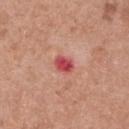workup: total-body-photography surveillance lesion; no biopsy | automated metrics: roughly 14 lightness units darker than nearby skin and a normalized lesion–skin contrast near 9.5; a border-irregularity index near 1.5/10, internal color variation of about 3 on a 0–10 scale, and radial color variation of about 1; a nevus-likeness score of about 0/100 and a detector confidence of about 100 out of 100 that the crop contains a lesion | image: ~15 mm tile from a whole-body skin photo | subject: female, roughly 60 years of age | size: ≈2.5 mm | site: the upper back.